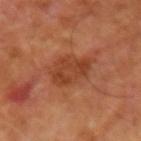Impression:
Part of a total-body skin-imaging series; this lesion was reviewed on a skin check and was not flagged for biopsy.
Image and clinical context:
A close-up tile cropped from a whole-body skin photograph, about 15 mm across. The total-body-photography lesion software estimated an average lesion color of about L≈41 a*≈27 b*≈35 (CIELAB), about 8 CIELAB-L* units darker than the surrounding skin, and a lesion-to-skin contrast of about 6.5 (normalized; higher = more distinct). Captured under cross-polarized illumination. From the right forearm. A male subject approximately 55 years of age. Measured at roughly 4.5 mm in maximum diameter.